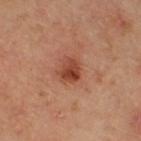<tbp_lesion>
<biopsy_status>not biopsied; imaged during a skin examination</biopsy_status>
<lighting>cross-polarized</lighting>
<lesion_size>
  <long_diameter_mm_approx>3.0</long_diameter_mm_approx>
</lesion_size>
<site>right thigh</site>
<image>
  <source>total-body photography crop</source>
  <field_of_view_mm>15</field_of_view_mm>
</image>
<automated_metrics>
  <border_irregularity_0_10>2.0</border_irregularity_0_10>
  <color_variation_0_10>6.0</color_variation_0_10>
  <peripheral_color_asymmetry>2.5</peripheral_color_asymmetry>
</automated_metrics>
<patient>
  <sex>male</sex>
  <age_approx>65</age_approx>
</patient>
</tbp_lesion>